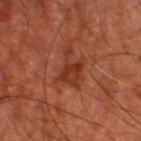Impression: No biopsy was performed on this lesion — it was imaged during a full skin examination and was not determined to be concerning. Image and clinical context: Longest diameter approximately 4.5 mm. The lesion-visualizer software estimated a footprint of about 8.5 mm², an outline eccentricity of about 0.75 (0 = round, 1 = elongated), and two-axis asymmetry of about 0.4. The analysis additionally found an average lesion color of about L≈36 a*≈29 b*≈32 (CIELAB), about 8 CIELAB-L* units darker than the surrounding skin, and a normalized border contrast of about 7. The subject is in their mid- to late 60s. The lesion is on the right thigh. A close-up tile cropped from a whole-body skin photograph, about 15 mm across.